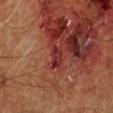Q: Was a biopsy performed?
A: catalogued during a skin exam; not biopsied
Q: Lesion location?
A: the left lower leg
Q: What kind of image is this?
A: 15 mm crop, total-body photography
Q: What is the lesion's diameter?
A: ~2.5 mm (longest diameter)
Q: Who is the patient?
A: male, aged 58–62